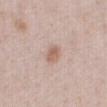Assessment: Part of a total-body skin-imaging series; this lesion was reviewed on a skin check and was not flagged for biopsy. Background: A 15 mm close-up extracted from a 3D total-body photography capture. From the chest. The lesion's longest dimension is about 2.5 mm. Captured under white-light illumination. A male patient, in their 60s.